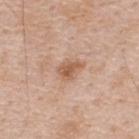  biopsy_status: not biopsied; imaged during a skin examination
  automated_metrics:
    area_mm2_approx: 5.0
    eccentricity: 0.75
    shape_asymmetry: 0.3
    cielab_L: 58
    cielab_a: 21
    cielab_b: 32
    vs_skin_darker_L: 10.0
    border_irregularity_0_10: 3.0
    color_variation_0_10: 2.5
  lighting: white-light
  image:
    source: total-body photography crop
    field_of_view_mm: 15
  site: upper back
  patient:
    sex: male
    age_approx: 50
  lesion_size:
    long_diameter_mm_approx: 3.0